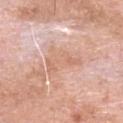follow-up: no biopsy performed (imaged during a skin exam) | acquisition: ~15 mm tile from a whole-body skin photo | subject: male, roughly 80 years of age | location: the head or neck | automated lesion analysis: an area of roughly 10 mm², an eccentricity of roughly 0.8, and a symmetry-axis asymmetry near 0.5; an average lesion color of about L≈67 a*≈21 b*≈30 (CIELAB), about 7 CIELAB-L* units darker than the surrounding skin, and a normalized lesion–skin contrast near 5.5 | size: about 5 mm.